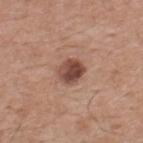Assessment:
Captured during whole-body skin photography for melanoma surveillance; the lesion was not biopsied.
Context:
A male subject approximately 55 years of age. Measured at roughly 3 mm in maximum diameter. From the upper back. An algorithmic analysis of the crop reported an area of roughly 6.5 mm², a shape eccentricity near 0.5, and a symmetry-axis asymmetry near 0.15. The software also gave an average lesion color of about L≈46 a*≈22 b*≈25 (CIELAB), about 15 CIELAB-L* units darker than the surrounding skin, and a normalized lesion–skin contrast near 10.5. The analysis additionally found an automated nevus-likeness rating near 75 out of 100 and a detector confidence of about 100 out of 100 that the crop contains a lesion. A roughly 15 mm field-of-view crop from a total-body skin photograph. Captured under white-light illumination.